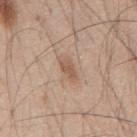Clinical impression: Recorded during total-body skin imaging; not selected for excision or biopsy. Image and clinical context: The lesion is located on the mid back. The lesion's longest dimension is about 3 mm. A male patient aged approximately 45. A roughly 15 mm field-of-view crop from a total-body skin photograph. The tile uses white-light illumination. Automated image analysis of the tile measured a lesion area of about 4.5 mm², a shape eccentricity near 0.85, and two-axis asymmetry of about 0.25. And it measured border irregularity of about 2.5 on a 0–10 scale, a color-variation rating of about 1.5/10, and peripheral color asymmetry of about 0.5.